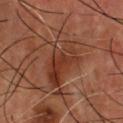biopsy status: imaged on a skin check; not biopsied | lighting: cross-polarized illumination | image-analysis metrics: a lesion color around L≈36 a*≈24 b*≈29 in CIELAB, about 8 CIELAB-L* units darker than the surrounding skin, and a normalized lesion–skin contrast near 7; a border-irregularity index near 4/10 | location: the chest | size: ~6 mm (longest diameter) | imaging modality: total-body-photography crop, ~15 mm field of view | patient: male, aged 53 to 57.